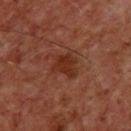No biopsy was performed on this lesion — it was imaged during a full skin examination and was not determined to be concerning. A male subject, aged around 60. A 15 mm close-up tile from a total-body photography series done for melanoma screening. From the front of the torso. Longest diameter approximately 3 mm.